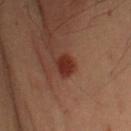Impression:
The lesion was tiled from a total-body skin photograph and was not biopsied.
Image and clinical context:
A male patient, in their mid- to late 50s. This image is a 15 mm lesion crop taken from a total-body photograph. Captured under cross-polarized illumination. From the left upper arm. Automated image analysis of the tile measured a footprint of about 4 mm², an eccentricity of roughly 0.75, and two-axis asymmetry of about 0.15. The analysis additionally found a color-variation rating of about 1.5/10. The software also gave a classifier nevus-likeness of about 90/100.